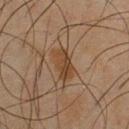<tbp_lesion>
<lesion_size>
  <long_diameter_mm_approx>3.5</long_diameter_mm_approx>
</lesion_size>
<image>
  <source>total-body photography crop</source>
  <field_of_view_mm>15</field_of_view_mm>
</image>
<patient>
  <sex>male</sex>
  <age_approx>45</age_approx>
</patient>
<site>chest</site>
<lighting>cross-polarized</lighting>
</tbp_lesion>